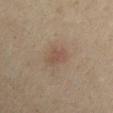Captured during whole-body skin photography for melanoma surveillance; the lesion was not biopsied. The lesion is on the right upper arm. The subject is a male aged approximately 50. This image is a 15 mm lesion crop taken from a total-body photograph. Imaged with cross-polarized lighting. The recorded lesion diameter is about 3 mm.